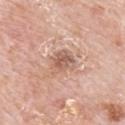Assessment:
The lesion was tiled from a total-body skin photograph and was not biopsied.
Context:
On the back. Captured under white-light illumination. A male patient aged approximately 80. This image is a 15 mm lesion crop taken from a total-body photograph.